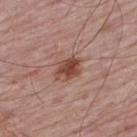<case>
<biopsy_status>not biopsied; imaged during a skin examination</biopsy_status>
<site>upper back</site>
<lesion_size>
  <long_diameter_mm_approx>3.0</long_diameter_mm_approx>
</lesion_size>
<patient>
  <sex>male</sex>
  <age_approx>65</age_approx>
</patient>
<automated_metrics>
  <lesion_detection_confidence_0_100>100</lesion_detection_confidence_0_100>
</automated_metrics>
<image>
  <source>total-body photography crop</source>
  <field_of_view_mm>15</field_of_view_mm>
</image>
</case>